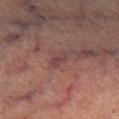Clinical impression:
Part of a total-body skin-imaging series; this lesion was reviewed on a skin check and was not flagged for biopsy.
Clinical summary:
The lesion's longest dimension is about 2.5 mm. This image is a 15 mm lesion crop taken from a total-body photograph. This is a cross-polarized tile. The lesion is located on the right lower leg. A male subject, approximately 75 years of age.